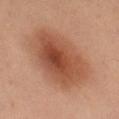{"biopsy_status": "not biopsied; imaged during a skin examination", "site": "left thigh", "patient": {"sex": "female", "age_approx": 40}, "lesion_size": {"long_diameter_mm_approx": 9.0}, "automated_metrics": {"vs_skin_contrast_norm": 8.5, "border_irregularity_0_10": 2.0}, "image": {"source": "total-body photography crop", "field_of_view_mm": 15}, "lighting": "cross-polarized"}